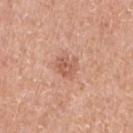The lesion was tiled from a total-body skin photograph and was not biopsied. Captured under white-light illumination. This image is a 15 mm lesion crop taken from a total-body photograph. A female subject, aged 68 to 72. On the arm. Approximately 2.5 mm at its widest.